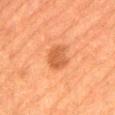The patient is a male approximately 85 years of age or older.
Located on the lower back.
A 15 mm close-up tile from a total-body photography series done for melanoma screening.